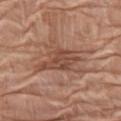Imaged during a routine full-body skin examination; the lesion was not biopsied and no histopathology is available. A close-up tile cropped from a whole-body skin photograph, about 15 mm across. The subject is a female roughly 75 years of age. On the left thigh. The recorded lesion diameter is about 6 mm.